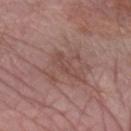{
  "biopsy_status": "not biopsied; imaged during a skin examination",
  "image": {
    "source": "total-body photography crop",
    "field_of_view_mm": 15
  },
  "patient": {
    "sex": "female",
    "age_approx": 65
  },
  "automated_metrics": {
    "area_mm2_approx": 4.0,
    "eccentricity": 0.95,
    "shape_asymmetry": 0.45,
    "cielab_L": 47,
    "cielab_a": 20,
    "cielab_b": 23,
    "vs_skin_darker_L": 6.0,
    "vs_skin_contrast_norm": 5.0,
    "border_irregularity_0_10": 5.0,
    "peripheral_color_asymmetry": 0.5,
    "lesion_detection_confidence_0_100": 95
  },
  "site": "arm"
}